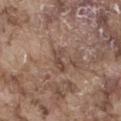Impression:
Captured during whole-body skin photography for melanoma surveillance; the lesion was not biopsied.
Clinical summary:
Captured under white-light illumination. The lesion is on the right thigh. A male patient aged around 75. A 15 mm close-up extracted from a 3D total-body photography capture. Measured at roughly 3 mm in maximum diameter.